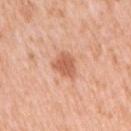Clinical impression:
The lesion was photographed on a routine skin check and not biopsied; there is no pathology result.
Background:
A close-up tile cropped from a whole-body skin photograph, about 15 mm across. The lesion is located on the arm. A female patient, aged approximately 40. Longest diameter approximately 3.5 mm. The lesion-visualizer software estimated a footprint of about 6.5 mm², an eccentricity of roughly 0.6, and two-axis asymmetry of about 0.25. It also reported border irregularity of about 2.5 on a 0–10 scale, a within-lesion color-variation index near 2/10, and a peripheral color-asymmetry measure near 0.5. Imaged with white-light lighting.